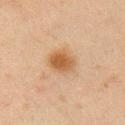biopsy status — no biopsy performed (imaged during a skin exam)
automated lesion analysis — a mean CIELAB color near L≈47 a*≈18 b*≈33 and a lesion–skin lightness drop of about 9
anatomic site — the right upper arm
subject — male, approximately 45 years of age
diameter — ≈3.5 mm
lighting — cross-polarized
image — ~15 mm tile from a whole-body skin photo